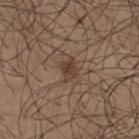Captured during whole-body skin photography for melanoma surveillance; the lesion was not biopsied. A male patient, aged around 25. Cropped from a total-body skin-imaging series; the visible field is about 15 mm. The tile uses white-light illumination. Located on the upper back. Automated image analysis of the tile measured a lesion area of about 4 mm², an eccentricity of roughly 0.7, and a shape-asymmetry score of about 0.25 (0 = symmetric). The software also gave border irregularity of about 2.5 on a 0–10 scale, internal color variation of about 3.5 on a 0–10 scale, and a peripheral color-asymmetry measure near 1.5.